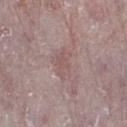workup: catalogued during a skin exam; not biopsied
imaging modality: ~15 mm tile from a whole-body skin photo
subject: female, roughly 65 years of age
site: the left lower leg